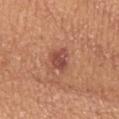workup: imaged on a skin check; not biopsied